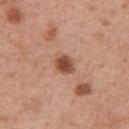Impression: Recorded during total-body skin imaging; not selected for excision or biopsy. Background: The tile uses white-light illumination. Cropped from a whole-body photographic skin survey; the tile spans about 15 mm. Automated tile analysis of the lesion measured a lesion area of about 4.5 mm² and a shape eccentricity near 0.6. The analysis additionally found internal color variation of about 3 on a 0–10 scale and peripheral color asymmetry of about 1. The software also gave a nevus-likeness score of about 95/100. The recorded lesion diameter is about 2.5 mm. On the right upper arm. The patient is a female in their 40s.